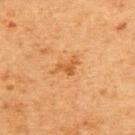notes: imaged on a skin check; not biopsied | subject: female, approximately 50 years of age | imaging modality: total-body-photography crop, ~15 mm field of view | site: the upper back | TBP lesion metrics: a lesion color around L≈49 a*≈23 b*≈40 in CIELAB and roughly 8 lightness units darker than nearby skin; border irregularity of about 5.5 on a 0–10 scale and radial color variation of about 0; an automated nevus-likeness rating near 0 out of 100 and lesion-presence confidence of about 100/100 | diameter: about 3 mm | lighting: cross-polarized.